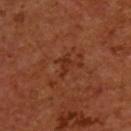follow-up = total-body-photography surveillance lesion; no biopsy
patient = male, about 55 years old
image source = ~15 mm crop, total-body skin-cancer survey
body site = the upper back